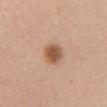anatomic site=the chest | patient=female, aged around 40 | image=15 mm crop, total-body photography.